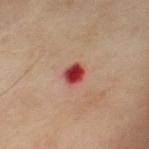This lesion was catalogued during total-body skin photography and was not selected for biopsy. A male patient roughly 70 years of age. From the chest. A region of skin cropped from a whole-body photographic capture, roughly 15 mm wide.